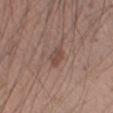Imaged during a routine full-body skin examination; the lesion was not biopsied and no histopathology is available.
Automated tile analysis of the lesion measured a lesion color around L≈46 a*≈18 b*≈24 in CIELAB and a lesion–skin lightness drop of about 8. The software also gave a border-irregularity rating of about 2.5/10 and a within-lesion color-variation index near 2.5/10. And it measured a classifier nevus-likeness of about 35/100 and a detector confidence of about 100 out of 100 that the crop contains a lesion.
A lesion tile, about 15 mm wide, cut from a 3D total-body photograph.
A male patient, aged 43 to 47.
Located on the left forearm.